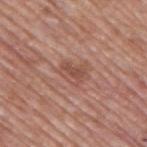Q: Is there a histopathology result?
A: total-body-photography surveillance lesion; no biopsy
Q: What are the patient's age and sex?
A: male, approximately 70 years of age
Q: Where on the body is the lesion?
A: the back
Q: What kind of image is this?
A: total-body-photography crop, ~15 mm field of view